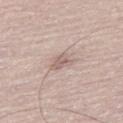Q: Was a biopsy performed?
A: total-body-photography surveillance lesion; no biopsy
Q: How large is the lesion?
A: about 3 mm
Q: Lesion location?
A: the right thigh
Q: Who is the patient?
A: male, aged 63 to 67
Q: What is the imaging modality?
A: ~15 mm crop, total-body skin-cancer survey
Q: What did automated image analysis measure?
A: a footprint of about 4 mm² and an eccentricity of roughly 0.75; a mean CIELAB color near L≈61 a*≈16 b*≈21, roughly 9 lightness units darker than nearby skin, and a normalized lesion–skin contrast near 6; a border-irregularity rating of about 3.5/10 and a within-lesion color-variation index near 3/10; an automated nevus-likeness rating near 0 out of 100 and a lesion-detection confidence of about 60/100